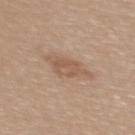workup — total-body-photography surveillance lesion; no biopsy
subject — female, in their 30s
lesion size — about 5 mm
imaging modality — ~15 mm tile from a whole-body skin photo
site — the back